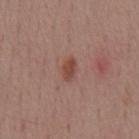follow-up: imaged on a skin check; not biopsied
anatomic site: the back
image-analysis metrics: a mean CIELAB color near L≈46 a*≈22 b*≈26, about 9 CIELAB-L* units darker than the surrounding skin, and a lesion-to-skin contrast of about 7.5 (normalized; higher = more distinct); an automated nevus-likeness rating near 90 out of 100
diameter: about 2.5 mm
imaging modality: 15 mm crop, total-body photography
tile lighting: white-light
subject: male, about 55 years old